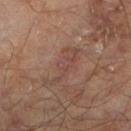workup: total-body-photography surveillance lesion; no biopsy
lesion size: about 5.5 mm
site: the left lower leg
image: ~15 mm crop, total-body skin-cancer survey
patient: male, aged around 65
lighting: cross-polarized
TBP lesion metrics: an average lesion color of about L≈43 a*≈19 b*≈23 (CIELAB), about 6 CIELAB-L* units darker than the surrounding skin, and a lesion-to-skin contrast of about 4.5 (normalized; higher = more distinct); a nevus-likeness score of about 0/100 and lesion-presence confidence of about 95/100